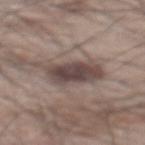Q: Was this lesion biopsied?
A: total-body-photography surveillance lesion; no biopsy
Q: How was this image acquired?
A: 15 mm crop, total-body photography
Q: What are the patient's age and sex?
A: male, aged 68–72
Q: Lesion location?
A: the back